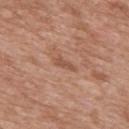<lesion>
  <biopsy_status>not biopsied; imaged during a skin examination</biopsy_status>
  <automated_metrics>
    <nevus_likeness_0_100>0</nevus_likeness_0_100>
    <lesion_detection_confidence_0_100>100</lesion_detection_confidence_0_100>
  </automated_metrics>
  <site>mid back</site>
  <patient>
    <sex>male</sex>
    <age_approx>50</age_approx>
  </patient>
  <lesion_size>
    <long_diameter_mm_approx>3.0</long_diameter_mm_approx>
  </lesion_size>
  <image>
    <source>total-body photography crop</source>
    <field_of_view_mm>15</field_of_view_mm>
  </image>
</lesion>